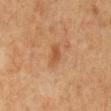- patient: male, about 60 years old
- location: the mid back
- acquisition: ~15 mm tile from a whole-body skin photo